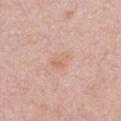Clinical impression: Recorded during total-body skin imaging; not selected for excision or biopsy. Image and clinical context: The patient is a female aged 28–32. Located on the chest. This is a white-light tile. The lesion's longest dimension is about 2.5 mm. A 15 mm close-up extracted from a 3D total-body photography capture.